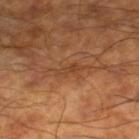Clinical summary: An algorithmic analysis of the crop reported a lesion area of about 2.5 mm², an outline eccentricity of about 0.9 (0 = round, 1 = elongated), and a symmetry-axis asymmetry near 0.4. The software also gave a lesion color around L≈41 a*≈22 b*≈33 in CIELAB. The analysis additionally found border irregularity of about 4.5 on a 0–10 scale, internal color variation of about 0 on a 0–10 scale, and peripheral color asymmetry of about 0. The tile uses cross-polarized illumination. A male subject about 60 years old. The lesion's longest dimension is about 2.5 mm. A roughly 15 mm field-of-view crop from a total-body skin photograph. On the right lower leg.